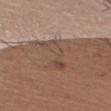Impression: Recorded during total-body skin imaging; not selected for excision or biopsy. Acquisition and patient details: Located on the right upper arm. A region of skin cropped from a whole-body photographic capture, roughly 15 mm wide. The subject is a male approximately 45 years of age.